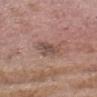Clinical impression:
The lesion was photographed on a routine skin check and not biopsied; there is no pathology result.
Image and clinical context:
The lesion's longest dimension is about 3 mm. The tile uses white-light illumination. From the head or neck. A male subject approximately 75 years of age. A 15 mm close-up extracted from a 3D total-body photography capture.